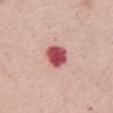Case summary:
* workup: total-body-photography surveillance lesion; no biopsy
* acquisition: 15 mm crop, total-body photography
* anatomic site: the chest
* size: ~3 mm (longest diameter)
* subject: female, roughly 60 years of age
* lighting: white-light